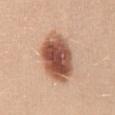Case summary:
• biopsy status · imaged on a skin check; not biopsied
• imaging modality · ~15 mm crop, total-body skin-cancer survey
• location · the back
• subject · female, approximately 30 years of age